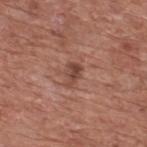notes: total-body-photography surveillance lesion; no biopsy
lesion size: about 3 mm
acquisition: 15 mm crop, total-body photography
patient: male, in their mid-70s
automated metrics: a nevus-likeness score of about 0/100
body site: the back
illumination: white-light illumination